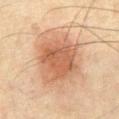This lesion was catalogued during total-body skin photography and was not selected for biopsy.
Located on the front of the torso.
A male patient aged 63–67.
The tile uses cross-polarized illumination.
A close-up tile cropped from a whole-body skin photograph, about 15 mm across.